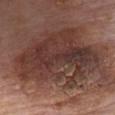This lesion was catalogued during total-body skin photography and was not selected for biopsy. The tile uses white-light illumination. Cropped from a total-body skin-imaging series; the visible field is about 15 mm. Located on the chest. The subject is a male in their 80s. An algorithmic analysis of the crop reported a mean CIELAB color near L≈36 a*≈19 b*≈22 and about 11 CIELAB-L* units darker than the surrounding skin. The software also gave a border-irregularity rating of about 4/10 and a within-lesion color-variation index near 6.5/10. The software also gave a lesion-detection confidence of about 100/100.